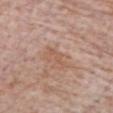{
  "biopsy_status": "not biopsied; imaged during a skin examination",
  "image": {
    "source": "total-body photography crop",
    "field_of_view_mm": 15
  },
  "patient": {
    "sex": "male",
    "age_approx": 75
  },
  "lesion_size": {
    "long_diameter_mm_approx": 3.0
  },
  "lighting": "white-light",
  "site": "chest"
}